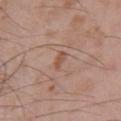Q: Was a biopsy performed?
A: total-body-photography surveillance lesion; no biopsy
Q: What are the patient's age and sex?
A: male, in their mid-60s
Q: What kind of image is this?
A: 15 mm crop, total-body photography
Q: Lesion location?
A: the chest